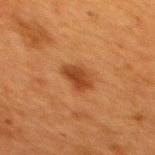Case summary:
* workup — imaged on a skin check; not biopsied
* subject — female, aged 38 to 42
* lighting — cross-polarized
* TBP lesion metrics — an area of roughly 5.5 mm², a shape eccentricity near 0.65, and a shape-asymmetry score of about 0.25 (0 = symmetric); a classifier nevus-likeness of about 95/100 and a lesion-detection confidence of about 100/100
* body site — the back
* imaging modality — ~15 mm crop, total-body skin-cancer survey
* lesion size — ~3 mm (longest diameter)